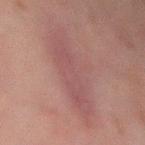Assessment: Imaged during a routine full-body skin examination; the lesion was not biopsied and no histopathology is available. Clinical summary: Cropped from a total-body skin-imaging series; the visible field is about 15 mm. The lesion's longest dimension is about 9 mm. Located on the left forearm. A male subject aged approximately 60. Imaged with cross-polarized lighting.